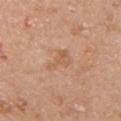Case summary:
– illumination: white-light illumination
– size: ≈3.5 mm
– image: ~15 mm tile from a whole-body skin photo
– subject: male, approximately 65 years of age
– location: the chest